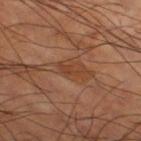Clinical impression: The lesion was photographed on a routine skin check and not biopsied; there is no pathology result. Acquisition and patient details: The recorded lesion diameter is about 3 mm. A male subject aged around 60. Located on the right thigh. Imaged with cross-polarized lighting. Automated image analysis of the tile measured a mean CIELAB color near L≈39 a*≈22 b*≈32 and about 7 CIELAB-L* units darker than the surrounding skin. It also reported a border-irregularity index near 8/10, a color-variation rating of about 0/10, and radial color variation of about 0. The software also gave an automated nevus-likeness rating near 0 out of 100 and a lesion-detection confidence of about 90/100. A close-up tile cropped from a whole-body skin photograph, about 15 mm across.